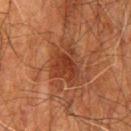– follow-up · imaged on a skin check; not biopsied
– TBP lesion metrics · an area of roughly 11 mm², an outline eccentricity of about 0.65 (0 = round, 1 = elongated), and a shape-asymmetry score of about 0.35 (0 = symmetric); a mean CIELAB color near L≈30 a*≈22 b*≈28, a lesion–skin lightness drop of about 8, and a normalized lesion–skin contrast near 7.5; peripheral color asymmetry of about 1
– subject · male, aged approximately 80
– body site · the mid back
– acquisition · ~15 mm tile from a whole-body skin photo
– illumination · cross-polarized